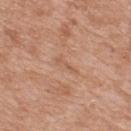| field | value |
|---|---|
| biopsy status | total-body-photography surveillance lesion; no biopsy |
| patient | male, approximately 50 years of age |
| image | total-body-photography crop, ~15 mm field of view |
| location | the mid back |
| TBP lesion metrics | an area of roughly 2.5 mm², an outline eccentricity of about 0.95 (0 = round, 1 = elongated), and a symmetry-axis asymmetry near 0.5; a border-irregularity rating of about 5.5/10, a color-variation rating of about 0/10, and a peripheral color-asymmetry measure near 0; a detector confidence of about 90 out of 100 that the crop contains a lesion |